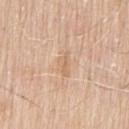workup: catalogued during a skin exam; not biopsied
lesion size: ≈3 mm
subject: male, aged 68–72
image source: ~15 mm tile from a whole-body skin photo
anatomic site: the arm
illumination: white-light